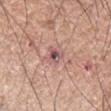This lesion was catalogued during total-body skin photography and was not selected for biopsy. A male patient about 65 years old. The lesion's longest dimension is about 2.5 mm. On the right forearm. Cropped from a whole-body photographic skin survey; the tile spans about 15 mm.